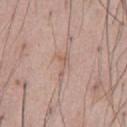– biopsy status: imaged on a skin check; not biopsied
– diameter: ~3.5 mm (longest diameter)
– patient: male, in their mid-50s
– TBP lesion metrics: a footprint of about 3.5 mm², an outline eccentricity of about 0.95 (0 = round, 1 = elongated), and two-axis asymmetry of about 0.65; a lesion color around L≈59 a*≈18 b*≈25 in CIELAB
– anatomic site: the front of the torso
– lighting: white-light
– acquisition: 15 mm crop, total-body photography